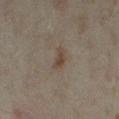Impression:
Imaged during a routine full-body skin examination; the lesion was not biopsied and no histopathology is available.
Clinical summary:
The lesion-visualizer software estimated border irregularity of about 4 on a 0–10 scale and radial color variation of about 0. It also reported a nevus-likeness score of about 65/100 and lesion-presence confidence of about 100/100. The lesion is located on the leg. A female patient approximately 35 years of age. Measured at roughly 2.5 mm in maximum diameter. A 15 mm crop from a total-body photograph taken for skin-cancer surveillance.